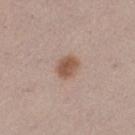<record>
  <biopsy_status>not biopsied; imaged during a skin examination</biopsy_status>
  <lesion_size>
    <long_diameter_mm_approx>3.0</long_diameter_mm_approx>
  </lesion_size>
  <image>
    <source>total-body photography crop</source>
    <field_of_view_mm>15</field_of_view_mm>
  </image>
  <lighting>white-light</lighting>
  <site>left thigh</site>
  <patient>
    <sex>female</sex>
    <age_approx>20</age_approx>
  </patient>
</record>